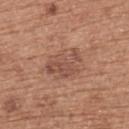workup=imaged on a skin check; not biopsied | image=~15 mm crop, total-body skin-cancer survey | patient=female, roughly 60 years of age | diameter=about 4.5 mm | location=the upper back.